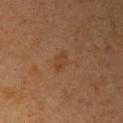Clinical impression:
The lesion was tiled from a total-body skin photograph and was not biopsied.
Image and clinical context:
Longest diameter approximately 2.5 mm. The tile uses cross-polarized illumination. The total-body-photography lesion software estimated a mean CIELAB color near L≈34 a*≈17 b*≈29 and about 4 CIELAB-L* units darker than the surrounding skin. The software also gave a nevus-likeness score of about 10/100 and a lesion-detection confidence of about 100/100. The lesion is on the right upper arm. A female patient, aged 48 to 52. A 15 mm close-up tile from a total-body photography series done for melanoma screening.